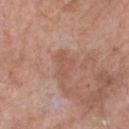Captured during whole-body skin photography for melanoma surveillance; the lesion was not biopsied. This image is a 15 mm lesion crop taken from a total-body photograph. The lesion is on the chest. The lesion-visualizer software estimated a border-irregularity rating of about 5.5/10, a within-lesion color-variation index near 0.5/10, and peripheral color asymmetry of about 0. The software also gave an automated nevus-likeness rating near 0 out of 100 and a lesion-detection confidence of about 100/100. A male patient about 60 years old.